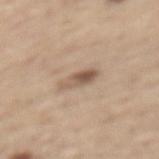Assessment:
Recorded during total-body skin imaging; not selected for excision or biopsy.
Context:
The recorded lesion diameter is about 3.5 mm. Cropped from a total-body skin-imaging series; the visible field is about 15 mm. The lesion is located on the back. A male patient, aged 68 to 72.